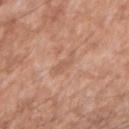biopsy_status: not biopsied; imaged during a skin examination
site: arm
image:
  source: total-body photography crop
  field_of_view_mm: 15
lighting: white-light
patient:
  sex: male
  age_approx: 55
automated_metrics:
  cielab_L: 58
  cielab_a: 22
  cielab_b: 31
  vs_skin_darker_L: 7.0
  vs_skin_contrast_norm: 4.5
  border_irregularity_0_10: 5.0
  color_variation_0_10: 0.5
  peripheral_color_asymmetry: 0.0
  nevus_likeness_0_100: 0
  lesion_detection_confidence_0_100: 100
lesion_size:
  long_diameter_mm_approx: 3.0